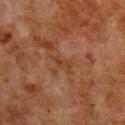The lesion was tiled from a total-body skin photograph and was not biopsied. From the right upper arm. Cropped from a total-body skin-imaging series; the visible field is about 15 mm. The subject is a male aged 78 to 82.